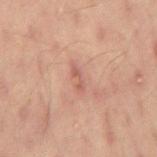workup — imaged on a skin check; not biopsied
image-analysis metrics — a footprint of about 2.5 mm², an outline eccentricity of about 0.95 (0 = round, 1 = elongated), and a shape-asymmetry score of about 0.45 (0 = symmetric); an average lesion color of about L≈53 a*≈23 b*≈26 (CIELAB), a lesion–skin lightness drop of about 8, and a normalized lesion–skin contrast near 6; a nevus-likeness score of about 0/100 and lesion-presence confidence of about 100/100
patient — male, aged approximately 60
size — about 3 mm
imaging modality — total-body-photography crop, ~15 mm field of view
lighting — cross-polarized
body site — the mid back